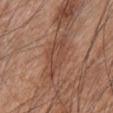workup: no biopsy performed (imaged during a skin exam) | lesion diameter: ~5 mm (longest diameter) | acquisition: 15 mm crop, total-body photography | image-analysis metrics: a lesion area of about 9.5 mm², an outline eccentricity of about 0.8 (0 = round, 1 = elongated), and a symmetry-axis asymmetry near 0.35; peripheral color asymmetry of about 1; a nevus-likeness score of about 0/100 | tile lighting: white-light illumination | anatomic site: the front of the torso | patient: male, in their mid-40s.